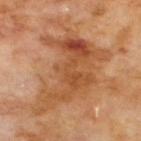Recorded during total-body skin imaging; not selected for excision or biopsy.